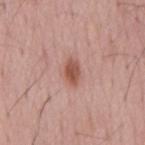Q: Is there a histopathology result?
A: catalogued during a skin exam; not biopsied
Q: Lesion size?
A: ≈3 mm
Q: Who is the patient?
A: male, in their mid-50s
Q: What lighting was used for the tile?
A: white-light illumination
Q: What kind of image is this?
A: total-body-photography crop, ~15 mm field of view